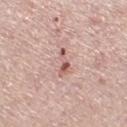{
  "biopsy_status": "not biopsied; imaged during a skin examination",
  "image": {
    "source": "total-body photography crop",
    "field_of_view_mm": 15
  },
  "lighting": "white-light",
  "patient": {
    "sex": "male",
    "age_approx": 60
  },
  "lesion_size": {
    "long_diameter_mm_approx": 3.5
  },
  "automated_metrics": {
    "border_irregularity_0_10": 6.0,
    "color_variation_0_10": 0.0,
    "peripheral_color_asymmetry": 0.0,
    "nevus_likeness_0_100": 0,
    "lesion_detection_confidence_0_100": 95
  },
  "site": "left lower leg"
}